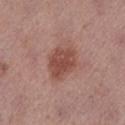The lesion was tiled from a total-body skin photograph and was not biopsied. The lesion is located on the left lower leg. The lesion-visualizer software estimated a lesion–skin lightness drop of about 10 and a lesion-to-skin contrast of about 8 (normalized; higher = more distinct). The analysis additionally found a border-irregularity rating of about 2/10, a within-lesion color-variation index near 2.5/10, and peripheral color asymmetry of about 1. A 15 mm close-up tile from a total-body photography series done for melanoma screening. A female subject, approximately 40 years of age. The lesion's longest dimension is about 4.5 mm.